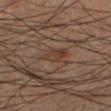notes: no biopsy performed (imaged during a skin exam)
body site: the leg
automated lesion analysis: an eccentricity of roughly 0.85 and a shape-asymmetry score of about 0.25 (0 = symmetric); an average lesion color of about L≈36 a*≈17 b*≈26 (CIELAB), about 6 CIELAB-L* units darker than the surrounding skin, and a lesion-to-skin contrast of about 6 (normalized; higher = more distinct); a border-irregularity rating of about 3/10, a within-lesion color-variation index near 2.5/10, and radial color variation of about 1
patient: male, roughly 60 years of age
image: 15 mm crop, total-body photography
lighting: cross-polarized illumination